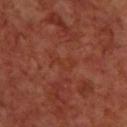biopsy status = imaged on a skin check; not biopsied | diameter = ~3.5 mm (longest diameter) | illumination = cross-polarized | subject = male, aged approximately 70 | site = the upper back | image = ~15 mm crop, total-body skin-cancer survey.